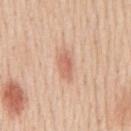Q: Was a biopsy performed?
A: total-body-photography surveillance lesion; no biopsy
Q: Who is the patient?
A: male, aged 58–62
Q: What did automated image analysis measure?
A: a mean CIELAB color near L≈64 a*≈23 b*≈31; border irregularity of about 3 on a 0–10 scale, a color-variation rating of about 2.5/10, and peripheral color asymmetry of about 1
Q: How was this image acquired?
A: ~15 mm tile from a whole-body skin photo
Q: How large is the lesion?
A: ≈4 mm
Q: Where on the body is the lesion?
A: the mid back
Q: How was the tile lit?
A: white-light